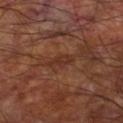The lesion was photographed on a routine skin check and not biopsied; there is no pathology result.